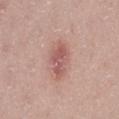Q: Is there a histopathology result?
A: total-body-photography surveillance lesion; no biopsy